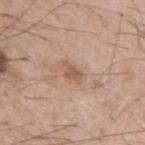Clinical impression: No biopsy was performed on this lesion — it was imaged during a full skin examination and was not determined to be concerning. Image and clinical context: The lesion-visualizer software estimated about 9 CIELAB-L* units darker than the surrounding skin and a normalized border contrast of about 6.5. It also reported a detector confidence of about 100 out of 100 that the crop contains a lesion. Cropped from a total-body skin-imaging series; the visible field is about 15 mm. Imaged with white-light lighting. A male patient aged 53–57. The lesion's longest dimension is about 3 mm.